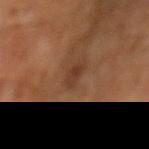Notes:
– biopsy status · total-body-photography surveillance lesion; no biopsy